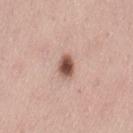follow-up: no biopsy performed (imaged during a skin exam)
body site: the left thigh
automated metrics: an area of roughly 4.5 mm², an outline eccentricity of about 0.65 (0 = round, 1 = elongated), and two-axis asymmetry of about 0.1; an average lesion color of about L≈53 a*≈21 b*≈25 (CIELAB) and about 18 CIELAB-L* units darker than the surrounding skin
tile lighting: white-light illumination
image source: total-body-photography crop, ~15 mm field of view
patient: female, about 40 years old